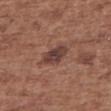workup = imaged on a skin check; not biopsied
subject = female, about 75 years old
acquisition = total-body-photography crop, ~15 mm field of view
site = the upper back
lesion diameter = ~3.5 mm (longest diameter)
illumination = white-light illumination